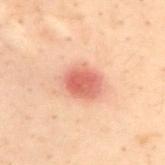Recorded during total-body skin imaging; not selected for excision or biopsy. The patient is a male roughly 30 years of age. From the upper back. Automated image analysis of the tile measured an average lesion color of about L≈52 a*≈25 b*≈27 (CIELAB), about 12 CIELAB-L* units darker than the surrounding skin, and a normalized border contrast of about 8. The software also gave an automated nevus-likeness rating near 100 out of 100 and lesion-presence confidence of about 100/100. Measured at roughly 4 mm in maximum diameter. Captured under cross-polarized illumination. A 15 mm close-up tile from a total-body photography series done for melanoma screening.